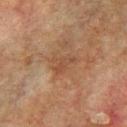Case summary:
– image source · total-body-photography crop, ~15 mm field of view
– lighting · cross-polarized
– image-analysis metrics · an area of roughly 5 mm², an eccentricity of roughly 0.8, and a shape-asymmetry score of about 0.45 (0 = symmetric); an automated nevus-likeness rating near 0 out of 100 and a detector confidence of about 100 out of 100 that the crop contains a lesion
– location · the left upper arm
– lesion size · ~3 mm (longest diameter)
– subject · male, aged around 75